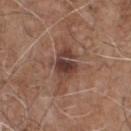workup — no biopsy performed (imaged during a skin exam) | body site — the chest | automated lesion analysis — an average lesion color of about L≈40 a*≈19 b*≈24 (CIELAB), a lesion–skin lightness drop of about 11, and a lesion-to-skin contrast of about 9 (normalized; higher = more distinct) | patient — male, aged approximately 70 | acquisition — 15 mm crop, total-body photography | lighting — white-light.